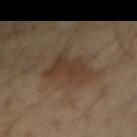biopsy status=catalogued during a skin exam; not biopsied
location=the right forearm
diameter=~5.5 mm (longest diameter)
subject=male, aged around 45
image=~15 mm tile from a whole-body skin photo
tile lighting=cross-polarized illumination